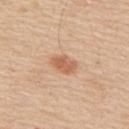  biopsy_status: not biopsied; imaged during a skin examination
  site: upper back
  patient:
    sex: male
    age_approx: 60
  image:
    source: total-body photography crop
    field_of_view_mm: 15
  lesion_size:
    long_diameter_mm_approx: 3.0
  lighting: white-light